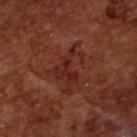Assessment: Part of a total-body skin-imaging series; this lesion was reviewed on a skin check and was not flagged for biopsy. Background: A close-up tile cropped from a whole-body skin photograph, about 15 mm across. A male subject about 65 years old. Captured under cross-polarized illumination.